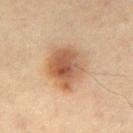Assessment: Imaged during a routine full-body skin examination; the lesion was not biopsied and no histopathology is available. Acquisition and patient details: Cropped from a total-body skin-imaging series; the visible field is about 15 mm. On the right thigh. Measured at roughly 5 mm in maximum diameter. A female patient roughly 45 years of age. The tile uses cross-polarized illumination.